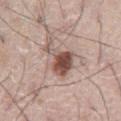Q: Was this lesion biopsied?
A: imaged on a skin check; not biopsied